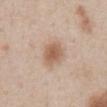Findings:
• workup: no biopsy performed (imaged during a skin exam)
• image: total-body-photography crop, ~15 mm field of view
• automated metrics: an outline eccentricity of about 0.65 (0 = round, 1 = elongated) and a shape-asymmetry score of about 0.15 (0 = symmetric); a mean CIELAB color near L≈60 a*≈18 b*≈30
• diameter: about 4 mm
• location: the abdomen
• lighting: white-light illumination
• patient: male, aged 58–62